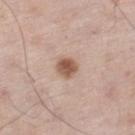follow-up: catalogued during a skin exam; not biopsied
imaging modality: 15 mm crop, total-body photography
patient: male, roughly 60 years of age
body site: the right lower leg
lighting: white-light
lesion diameter: ≈2.5 mm
TBP lesion metrics: a footprint of about 5 mm² and a shape-asymmetry score of about 0.2 (0 = symmetric); an average lesion color of about L≈56 a*≈20 b*≈28 (CIELAB), roughly 14 lightness units darker than nearby skin, and a normalized border contrast of about 9; a nevus-likeness score of about 95/100 and lesion-presence confidence of about 100/100